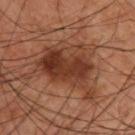Captured during whole-body skin photography for melanoma surveillance; the lesion was not biopsied.
A 15 mm crop from a total-body photograph taken for skin-cancer surveillance.
From the upper back.
A male patient in their 60s.